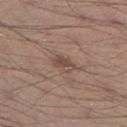Assessment:
Recorded during total-body skin imaging; not selected for excision or biopsy.
Clinical summary:
On the leg. A 15 mm close-up extracted from a 3D total-body photography capture. A male patient aged 28–32.